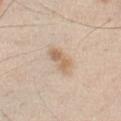Notes:
– follow-up — imaged on a skin check; not biopsied
– automated metrics — a footprint of about 5.5 mm², a shape eccentricity near 0.85, and a shape-asymmetry score of about 0.25 (0 = symmetric)
– site — the chest
– patient — male, aged 43 to 47
– lighting — white-light
– image source — 15 mm crop, total-body photography